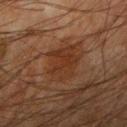Clinical impression:
No biopsy was performed on this lesion — it was imaged during a full skin examination and was not determined to be concerning.
Context:
An algorithmic analysis of the crop reported an area of roughly 14 mm² and a shape eccentricity near 0.65. It also reported a mean CIELAB color near L≈26 a*≈17 b*≈26, a lesion–skin lightness drop of about 5, and a normalized border contrast of about 6.5. A lesion tile, about 15 mm wide, cut from a 3D total-body photograph. The tile uses cross-polarized illumination. Located on the right upper arm. A male subject, about 65 years old. The lesion's longest dimension is about 5 mm.